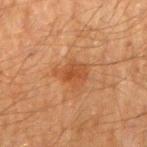| feature | finding |
|---|---|
| notes | catalogued during a skin exam; not biopsied |
| lesion size | ~4 mm (longest diameter) |
| acquisition | total-body-photography crop, ~15 mm field of view |
| location | the right upper arm |
| subject | male, in their mid-60s |
| illumination | cross-polarized |